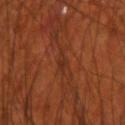The lesion was photographed on a routine skin check and not biopsied; there is no pathology result. Imaged with cross-polarized lighting. A region of skin cropped from a whole-body photographic capture, roughly 15 mm wide. About 2.5 mm across. Automated image analysis of the tile measured an area of roughly 2.5 mm² and an outline eccentricity of about 0.9 (0 = round, 1 = elongated). And it measured roughly 5 lightness units darker than nearby skin and a normalized border contrast of about 5.5. The analysis additionally found a lesion-detection confidence of about 85/100. Located on the right upper arm. A male patient, about 70 years old.